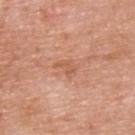biopsy status: no biopsy performed (imaged during a skin exam)
image source: 15 mm crop, total-body photography
illumination: white-light
patient: male, approximately 60 years of age
body site: the upper back
diameter: ~2.5 mm (longest diameter)